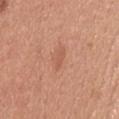{
  "biopsy_status": "not biopsied; imaged during a skin examination",
  "lighting": "white-light",
  "automated_metrics": {
    "eccentricity": 0.95,
    "shape_asymmetry": 0.25,
    "border_irregularity_0_10": 3.0,
    "color_variation_0_10": 0.0,
    "peripheral_color_asymmetry": 0.0,
    "lesion_detection_confidence_0_100": 100
  },
  "site": "head or neck",
  "image": {
    "source": "total-body photography crop",
    "field_of_view_mm": 15
  },
  "patient": {
    "sex": "male",
    "age_approx": 30
  },
  "lesion_size": {
    "long_diameter_mm_approx": 3.0
  }
}